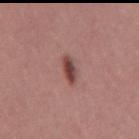Findings:
– biopsy status — catalogued during a skin exam; not biopsied